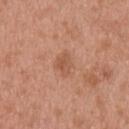The lesion was tiled from a total-body skin photograph and was not biopsied. Captured under white-light illumination. Measured at roughly 2.5 mm in maximum diameter. The lesion is on the back. Cropped from a whole-body photographic skin survey; the tile spans about 15 mm. A male subject aged approximately 30.